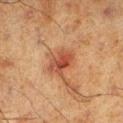Findings:
– image-analysis metrics · a lesion color around L≈39 a*≈22 b*≈28 in CIELAB, about 9 CIELAB-L* units darker than the surrounding skin, and a lesion-to-skin contrast of about 7.5 (normalized; higher = more distinct); a border-irregularity index near 2/10, a within-lesion color-variation index near 5.5/10, and a peripheral color-asymmetry measure near 2; a detector confidence of about 100 out of 100 that the crop contains a lesion
– illumination · cross-polarized
– imaging modality · ~15 mm tile from a whole-body skin photo
– patient · male, approximately 60 years of age
– anatomic site · the left lower leg
– lesion diameter · ≈4 mm